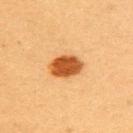Assessment: The lesion was photographed on a routine skin check and not biopsied; there is no pathology result. Acquisition and patient details: Cropped from a total-body skin-imaging series; the visible field is about 15 mm. A female subject aged 18 to 22. This is a cross-polarized tile. Measured at roughly 4 mm in maximum diameter. Located on the upper back.